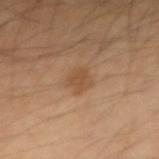Findings:
• notes — total-body-photography surveillance lesion; no biopsy
• automated metrics — a shape eccentricity near 0.4 and two-axis asymmetry of about 0.25; an average lesion color of about L≈49 a*≈19 b*≈33 (CIELAB), a lesion–skin lightness drop of about 7, and a lesion-to-skin contrast of about 6 (normalized; higher = more distinct); an automated nevus-likeness rating near 35 out of 100
• patient — male, aged 48–52
• image — total-body-photography crop, ~15 mm field of view
• tile lighting — cross-polarized
• site — the left arm
• diameter — about 2.5 mm